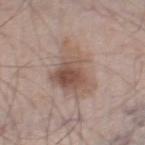The lesion was tiled from a total-body skin photograph and was not biopsied. About 7 mm across. A male patient, roughly 60 years of age. A lesion tile, about 15 mm wide, cut from a 3D total-body photograph. The lesion is on the leg.